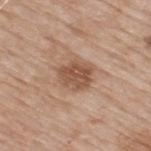* notes: imaged on a skin check; not biopsied
* subject: male, aged 63–67
* image: 15 mm crop, total-body photography
* illumination: white-light
* lesion diameter: ~4 mm (longest diameter)
* location: the upper back